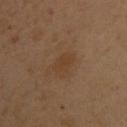Assessment:
The lesion was photographed on a routine skin check and not biopsied; there is no pathology result.
Image and clinical context:
The lesion is located on the upper back. The recorded lesion diameter is about 2.5 mm. The patient is a female aged around 40. Cropped from a whole-body photographic skin survey; the tile spans about 15 mm.